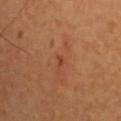notes: total-body-photography surveillance lesion; no biopsy
location: the upper back
automated lesion analysis: radial color variation of about 0
patient: male, aged 63–67
image source: ~15 mm crop, total-body skin-cancer survey
illumination: cross-polarized